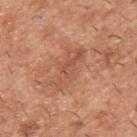Context: The total-body-photography lesion software estimated a footprint of about 9.5 mm² and an eccentricity of roughly 0.95. Cropped from a whole-body photographic skin survey; the tile spans about 15 mm. From the upper back. The lesion's longest dimension is about 6 mm. A male subject about 40 years old.